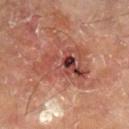Q: Was this lesion biopsied?
A: no biopsy performed (imaged during a skin exam)
Q: What did automated image analysis measure?
A: a mean CIELAB color near L≈46 a*≈27 b*≈28, about 11 CIELAB-L* units darker than the surrounding skin, and a lesion-to-skin contrast of about 8 (normalized; higher = more distinct)
Q: Patient demographics?
A: male, approximately 70 years of age
Q: Where on the body is the lesion?
A: the right lower leg
Q: How was the tile lit?
A: cross-polarized illumination
Q: How was this image acquired?
A: ~15 mm crop, total-body skin-cancer survey